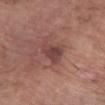| field | value |
|---|---|
| biopsy status | imaged on a skin check; not biopsied |
| patient | male, aged 58–62 |
| size | about 3.5 mm |
| acquisition | ~15 mm crop, total-body skin-cancer survey |
| automated metrics | a lesion color around L≈42 a*≈23 b*≈22 in CIELAB and a normalized border contrast of about 7.5; a border-irregularity index near 3.5/10 and a within-lesion color-variation index near 3/10; a classifier nevus-likeness of about 15/100 and a lesion-detection confidence of about 100/100 |
| lighting | white-light illumination |
| anatomic site | the right forearm |